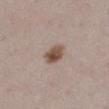{"site": "left lower leg", "lesion_size": {"long_diameter_mm_approx": 3.0}, "automated_metrics": {"cielab_L": 50, "cielab_a": 16, "cielab_b": 25, "vs_skin_darker_L": 14.0, "vs_skin_contrast_norm": 10.5, "color_variation_0_10": 5.0, "peripheral_color_asymmetry": 2.0, "nevus_likeness_0_100": 95}, "image": {"source": "total-body photography crop", "field_of_view_mm": 15}, "patient": {"sex": "female", "age_approx": 40}}